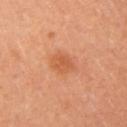Part of a total-body skin-imaging series; this lesion was reviewed on a skin check and was not flagged for biopsy. A female patient aged 33 to 37. The tile uses cross-polarized illumination. An algorithmic analysis of the crop reported an area of roughly 5.5 mm². About 3 mm across. A roughly 15 mm field-of-view crop from a total-body skin photograph. From the right upper arm.